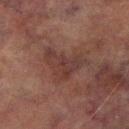notes: catalogued during a skin exam; not biopsied | anatomic site: the right lower leg | subject: male, approximately 75 years of age | diameter: about 5.5 mm | imaging modality: ~15 mm crop, total-body skin-cancer survey | illumination: cross-polarized.